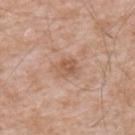Background:
From the chest. A male subject approximately 60 years of age. A region of skin cropped from a whole-body photographic capture, roughly 15 mm wide.